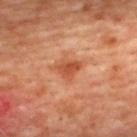Findings:
• workup — catalogued during a skin exam; not biopsied
• lighting — cross-polarized illumination
• site — the upper back
• image — ~15 mm tile from a whole-body skin photo
• patient — female, roughly 45 years of age